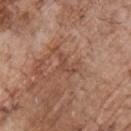biopsy_status: not biopsied; imaged during a skin examination
image:
  source: total-body photography crop
  field_of_view_mm: 15
lesion_size:
  long_diameter_mm_approx: 3.0
automated_metrics:
  area_mm2_approx: 3.0
  eccentricity: 0.9
  shape_asymmetry: 0.7
  nevus_likeness_0_100: 0
lighting: white-light
site: chest
patient:
  sex: male
  age_approx: 70